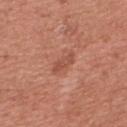Q: Was a biopsy performed?
A: catalogued during a skin exam; not biopsied
Q: Lesion location?
A: the front of the torso
Q: What kind of image is this?
A: ~15 mm crop, total-body skin-cancer survey
Q: How was the tile lit?
A: white-light
Q: Who is the patient?
A: male, about 45 years old
Q: What is the lesion's diameter?
A: ~3 mm (longest diameter)